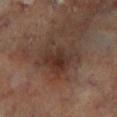Recorded during total-body skin imaging; not selected for excision or biopsy. The lesion's longest dimension is about 5.5 mm. A male patient, in their mid- to late 60s. The lesion is located on the left lower leg. Automated tile analysis of the lesion measured a border-irregularity rating of about 6.5/10, a color-variation rating of about 4.5/10, and peripheral color asymmetry of about 1.5. The tile uses cross-polarized illumination. Cropped from a total-body skin-imaging series; the visible field is about 15 mm.